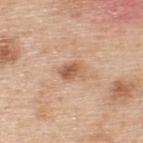Notes:
* notes — catalogued during a skin exam; not biopsied
* image-analysis metrics — a border-irregularity rating of about 2.5/10, a color-variation rating of about 5/10, and a peripheral color-asymmetry measure near 1.5; a nevus-likeness score of about 30/100 and a lesion-detection confidence of about 100/100
* diameter — ≈3 mm
* anatomic site — the upper back
* lighting — white-light
* image source — 15 mm crop, total-body photography
* patient — male, aged 48–52